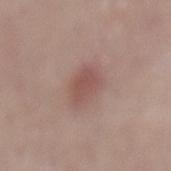The lesion is on the mid back.
A 15 mm crop from a total-body photograph taken for skin-cancer surveillance.
Imaged with white-light lighting.
The patient is a male roughly 70 years of age.